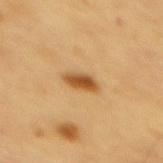- workup · imaged on a skin check; not biopsied
- image · total-body-photography crop, ~15 mm field of view
- subject · male, aged approximately 60
- anatomic site · the mid back